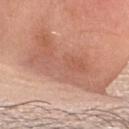{"biopsy_status": "not biopsied; imaged during a skin examination", "site": "head or neck", "patient": {"sex": "male", "age_approx": 35}, "automated_metrics": {"cielab_L": 59, "cielab_a": 24, "cielab_b": 30, "vs_skin_darker_L": 9.0, "vs_skin_contrast_norm": 6.0, "nevus_likeness_0_100": 0, "lesion_detection_confidence_0_100": 95}, "image": {"source": "total-body photography crop", "field_of_view_mm": 15}, "lighting": "white-light", "lesion_size": {"long_diameter_mm_approx": 10.5}}